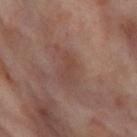Imaged with cross-polarized lighting.
The patient is a female roughly 55 years of age.
Located on the right thigh.
Measured at roughly 3 mm in maximum diameter.
Cropped from a whole-body photographic skin survey; the tile spans about 15 mm.